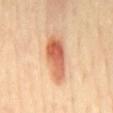Q: Is there a histopathology result?
A: catalogued during a skin exam; not biopsied
Q: Who is the patient?
A: male, aged 63–67
Q: What is the imaging modality?
A: ~15 mm crop, total-body skin-cancer survey
Q: Lesion location?
A: the mid back
Q: What did automated image analysis measure?
A: an area of roughly 14 mm², an eccentricity of roughly 0.9, and a shape-asymmetry score of about 0.25 (0 = symmetric); roughly 15 lightness units darker than nearby skin and a normalized border contrast of about 9; a classifier nevus-likeness of about 100/100 and a lesion-detection confidence of about 100/100